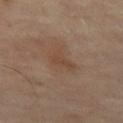follow-up — imaged on a skin check; not biopsied
image — total-body-photography crop, ~15 mm field of view
lesion diameter — ~3.5 mm (longest diameter)
anatomic site — the left thigh
patient — male, roughly 65 years of age
illumination — cross-polarized